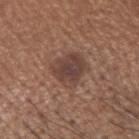• image-analysis metrics · a mean CIELAB color near L≈42 a*≈18 b*≈23, a lesion–skin lightness drop of about 9, and a lesion-to-skin contrast of about 8 (normalized; higher = more distinct)
• size · about 4 mm
• subject · male, in their mid- to late 60s
• image source · ~15 mm tile from a whole-body skin photo
• tile lighting · white-light illumination
• location · the head or neck